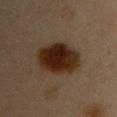Q: Was a biopsy performed?
A: no biopsy performed (imaged during a skin exam)
Q: Illumination type?
A: cross-polarized illumination
Q: Lesion size?
A: ≈6 mm
Q: What is the anatomic site?
A: the left upper arm
Q: What is the imaging modality?
A: total-body-photography crop, ~15 mm field of view
Q: Automated lesion metrics?
A: a footprint of about 19 mm², an eccentricity of roughly 0.75, and a shape-asymmetry score of about 0.1 (0 = symmetric); a lesion-to-skin contrast of about 15 (normalized; higher = more distinct); a lesion-detection confidence of about 100/100
Q: What are the patient's age and sex?
A: female, approximately 45 years of age